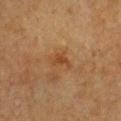Recorded during total-body skin imaging; not selected for excision or biopsy. Located on the chest. The lesion-visualizer software estimated a lesion area of about 3.5 mm², a shape eccentricity near 0.8, and a shape-asymmetry score of about 0.45 (0 = symmetric). The analysis additionally found border irregularity of about 4 on a 0–10 scale, internal color variation of about 2 on a 0–10 scale, and a peripheral color-asymmetry measure near 0.5. And it measured a classifier nevus-likeness of about 0/100 and a lesion-detection confidence of about 100/100. The patient is a female approximately 55 years of age. This image is a 15 mm lesion crop taken from a total-body photograph. This is a cross-polarized tile. Approximately 3 mm at its widest.